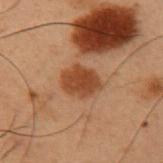Case summary:
– follow-up · catalogued during a skin exam; not biopsied
– lesion size · about 4 mm
– image-analysis metrics · a lesion area of about 9.5 mm², an eccentricity of roughly 0.5, and a symmetry-axis asymmetry near 0.15; a mean CIELAB color near L≈36 a*≈21 b*≈30 and a normalized lesion–skin contrast near 9; internal color variation of about 2 on a 0–10 scale and a peripheral color-asymmetry measure near 0.5
– anatomic site · the left upper arm
– image source · 15 mm crop, total-body photography
– subject · male, aged approximately 55